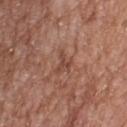<record>
  <biopsy_status>not biopsied; imaged during a skin examination</biopsy_status>
  <lighting>white-light</lighting>
  <site>upper back</site>
  <patient>
    <sex>female</sex>
    <age_approx>75</age_approx>
  </patient>
  <image>
    <source>total-body photography crop</source>
    <field_of_view_mm>15</field_of_view_mm>
  </image>
  <lesion_size>
    <long_diameter_mm_approx>3.0</long_diameter_mm_approx>
  </lesion_size>
</record>